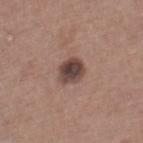Recorded during total-body skin imaging; not selected for excision or biopsy. The lesion is on the left lower leg. The patient is a male roughly 65 years of age. A region of skin cropped from a whole-body photographic capture, roughly 15 mm wide.